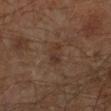The lesion was tiled from a total-body skin photograph and was not biopsied. About 2.5 mm across. From the left lower leg. Imaged with cross-polarized lighting. A close-up tile cropped from a whole-body skin photograph, about 15 mm across. The patient is a male aged 58 to 62.